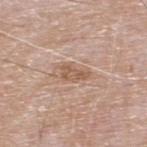follow-up: no biopsy performed (imaged during a skin exam) | size: ~3 mm (longest diameter) | location: the upper back | image source: ~15 mm tile from a whole-body skin photo | patient: male, aged 63 to 67 | TBP lesion metrics: a shape-asymmetry score of about 0.4 (0 = symmetric); a border-irregularity index near 4/10, internal color variation of about 2 on a 0–10 scale, and peripheral color asymmetry of about 0.5; a classifier nevus-likeness of about 0/100 and a lesion-detection confidence of about 100/100 | tile lighting: white-light illumination.